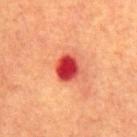Clinical impression:
Captured during whole-body skin photography for melanoma surveillance; the lesion was not biopsied.
Clinical summary:
The patient is a male aged approximately 65. The lesion's longest dimension is about 3 mm. The tile uses cross-polarized illumination. On the abdomen. A region of skin cropped from a whole-body photographic capture, roughly 15 mm wide.